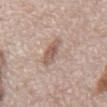patient:
  sex: male
  age_approx: 70
lighting: white-light
image:
  source: total-body photography crop
  field_of_view_mm: 15
site: abdomen
lesion_size:
  long_diameter_mm_approx: 4.5